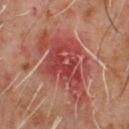Impression:
The lesion was photographed on a routine skin check and not biopsied; there is no pathology result.
Background:
The subject is a male aged around 60. The lesion is on the chest. Captured under cross-polarized illumination. The lesion-visualizer software estimated a lesion area of about 32 mm², an eccentricity of roughly 0.85, and a shape-asymmetry score of about 0.35 (0 = symmetric). The software also gave a classifier nevus-likeness of about 0/100 and a detector confidence of about 100 out of 100 that the crop contains a lesion. This image is a 15 mm lesion crop taken from a total-body photograph. The recorded lesion diameter is about 10 mm.